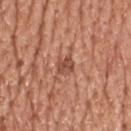{"lesion_size": {"long_diameter_mm_approx": 3.0}, "lighting": "white-light", "site": "head or neck", "patient": {"sex": "male", "age_approx": 70}, "image": {"source": "total-body photography crop", "field_of_view_mm": 15}}